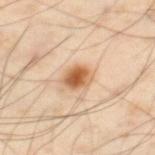Part of a total-body skin-imaging series; this lesion was reviewed on a skin check and was not flagged for biopsy.
From the left thigh.
A close-up tile cropped from a whole-body skin photograph, about 15 mm across.
A male subject about 55 years old.
The lesion's longest dimension is about 3 mm.